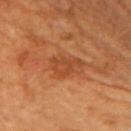Q: Is there a histopathology result?
A: catalogued during a skin exam; not biopsied
Q: Who is the patient?
A: female, roughly 60 years of age
Q: What is the anatomic site?
A: the left upper arm
Q: How was this image acquired?
A: total-body-photography crop, ~15 mm field of view